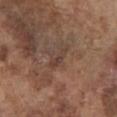Assessment: Imaged during a routine full-body skin examination; the lesion was not biopsied and no histopathology is available. Clinical summary: The patient is a male roughly 75 years of age. About 3 mm across. A 15 mm close-up tile from a total-body photography series done for melanoma screening. On the chest.